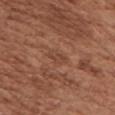This lesion was catalogued during total-body skin photography and was not selected for biopsy.